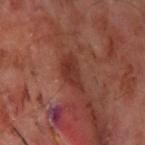The lesion was photographed on a routine skin check and not biopsied; there is no pathology result. The subject is a male roughly 65 years of age. The lesion is located on the left upper arm. The lesion-visualizer software estimated a lesion color around L≈33 a*≈26 b*≈26 in CIELAB and a normalized border contrast of about 7.5. It also reported border irregularity of about 4 on a 0–10 scale, a color-variation rating of about 2.5/10, and radial color variation of about 1. And it measured a nevus-likeness score of about 0/100 and a detector confidence of about 100 out of 100 that the crop contains a lesion. A lesion tile, about 15 mm wide, cut from a 3D total-body photograph.